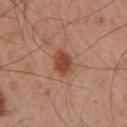Impression: Part of a total-body skin-imaging series; this lesion was reviewed on a skin check and was not flagged for biopsy. Clinical summary: The tile uses cross-polarized illumination. An algorithmic analysis of the crop reported a shape eccentricity near 0.75 and two-axis asymmetry of about 0.2. The software also gave an average lesion color of about L≈35 a*≈20 b*≈26 (CIELAB), a lesion–skin lightness drop of about 9, and a lesion-to-skin contrast of about 9 (normalized; higher = more distinct). Longest diameter approximately 3 mm. A male subject, approximately 45 years of age. A 15 mm close-up tile from a total-body photography series done for melanoma screening. From the right lower leg.